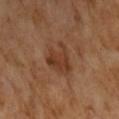Captured during whole-body skin photography for melanoma surveillance; the lesion was not biopsied.